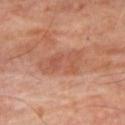No biopsy was performed on this lesion — it was imaged during a full skin examination and was not determined to be concerning. A male patient about 70 years old. Cropped from a total-body skin-imaging series; the visible field is about 15 mm. Longest diameter approximately 5.5 mm. This is a cross-polarized tile. Located on the leg.